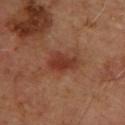follow-up=catalogued during a skin exam; not biopsied
patient=male, in their mid-60s
lesion diameter=~4 mm (longest diameter)
TBP lesion metrics=a border-irregularity index near 3/10 and internal color variation of about 2.5 on a 0–10 scale
anatomic site=the back
image=~15 mm tile from a whole-body skin photo